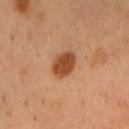– biopsy status · no biopsy performed (imaged during a skin exam)
– patient · male, aged 53 to 57
– location · the chest
– imaging modality · 15 mm crop, total-body photography